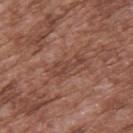| field | value |
|---|---|
| biopsy status | imaged on a skin check; not biopsied |
| anatomic site | the upper back |
| image | ~15 mm crop, total-body skin-cancer survey |
| patient | male, aged 73 to 77 |